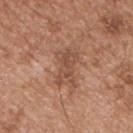Part of a total-body skin-imaging series; this lesion was reviewed on a skin check and was not flagged for biopsy. On the chest. A male patient in their mid- to late 50s. The total-body-photography lesion software estimated a detector confidence of about 100 out of 100 that the crop contains a lesion. A 15 mm crop from a total-body photograph taken for skin-cancer surveillance. This is a white-light tile. Measured at roughly 4 mm in maximum diameter.